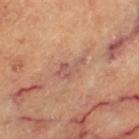biopsy status = no biopsy performed (imaged during a skin exam) | anatomic site = the left thigh | lesion diameter = ~3.5 mm (longest diameter) | TBP lesion metrics = an area of roughly 4.5 mm², a shape eccentricity near 0.85, and two-axis asymmetry of about 0.5; border irregularity of about 6 on a 0–10 scale and peripheral color asymmetry of about 0.5; a classifier nevus-likeness of about 0/100 | subject = female, aged approximately 60 | acquisition = ~15 mm crop, total-body skin-cancer survey | illumination = cross-polarized.